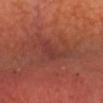Findings:
- follow-up · total-body-photography surveillance lesion; no biopsy
- imaging modality · ~15 mm crop, total-body skin-cancer survey
- subject · male, in their mid-50s
- location · the head or neck